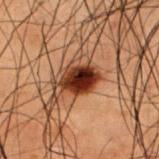Q: Is there a histopathology result?
A: total-body-photography surveillance lesion; no biopsy
Q: Lesion location?
A: the upper back
Q: How was this image acquired?
A: ~15 mm tile from a whole-body skin photo
Q: Patient demographics?
A: male, about 50 years old
Q: Illumination type?
A: cross-polarized illumination
Q: What is the lesion's diameter?
A: ~4 mm (longest diameter)